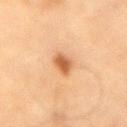Imaged during a routine full-body skin examination; the lesion was not biopsied and no histopathology is available.
On the right upper arm.
A 15 mm crop from a total-body photograph taken for skin-cancer surveillance.
A male patient aged approximately 65.